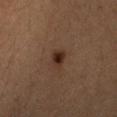{"biopsy_status": "not biopsied; imaged during a skin examination", "image": {"source": "total-body photography crop", "field_of_view_mm": 15}, "site": "upper back", "lesion_size": {"long_diameter_mm_approx": 3.0}, "patient": {"sex": "male", "age_approx": 30}}